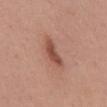Notes:
• biopsy status: no biopsy performed (imaged during a skin exam)
• acquisition: total-body-photography crop, ~15 mm field of view
• site: the mid back
• subject: female, aged approximately 50
• tile lighting: white-light